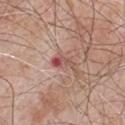| key | value |
|---|---|
| biopsy status | no biopsy performed (imaged during a skin exam) |
| automated metrics | peripheral color asymmetry of about 0.5 |
| size | ≈3 mm |
| subject | male, about 65 years old |
| location | the chest |
| acquisition | ~15 mm tile from a whole-body skin photo |